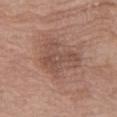Part of a total-body skin-imaging series; this lesion was reviewed on a skin check and was not flagged for biopsy.
About 5.5 mm across.
The lesion is on the right thigh.
The subject is a female aged approximately 70.
A 15 mm close-up extracted from a 3D total-body photography capture.